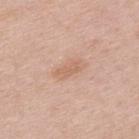{"biopsy_status": "not biopsied; imaged during a skin examination", "patient": {"sex": "male", "age_approx": 55}, "image": {"source": "total-body photography crop", "field_of_view_mm": 15}, "automated_metrics": {"border_irregularity_0_10": 4.5, "color_variation_0_10": 0.0, "peripheral_color_asymmetry": 0.0}, "lighting": "white-light", "site": "upper back"}